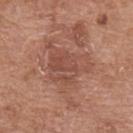Q: Was a biopsy performed?
A: no biopsy performed (imaged during a skin exam)
Q: What kind of image is this?
A: total-body-photography crop, ~15 mm field of view
Q: Lesion location?
A: the left upper arm
Q: What are the patient's age and sex?
A: male, approximately 80 years of age
Q: How was the tile lit?
A: white-light
Q: How large is the lesion?
A: ≈6 mm